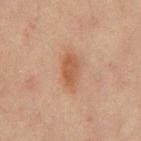Q: Was a biopsy performed?
A: no biopsy performed (imaged during a skin exam)
Q: What are the patient's age and sex?
A: male, in their 70s
Q: What is the anatomic site?
A: the mid back
Q: How was this image acquired?
A: 15 mm crop, total-body photography